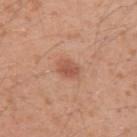{
  "biopsy_status": "not biopsied; imaged during a skin examination",
  "image": {
    "source": "total-body photography crop",
    "field_of_view_mm": 15
  },
  "patient": {
    "sex": "male",
    "age_approx": 30
  },
  "lesion_size": {
    "long_diameter_mm_approx": 2.5
  },
  "site": "left upper arm",
  "lighting": "white-light"
}